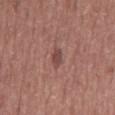No biopsy was performed on this lesion — it was imaged during a full skin examination and was not determined to be concerning. A male subject aged 58–62. The tile uses white-light illumination. A lesion tile, about 15 mm wide, cut from a 3D total-body photograph. The lesion is on the mid back. The lesion's longest dimension is about 2.5 mm.